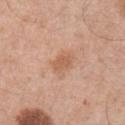Impression:
No biopsy was performed on this lesion — it was imaged during a full skin examination and was not determined to be concerning.
Background:
Imaged with white-light lighting. Automated image analysis of the tile measured a lesion area of about 3.5 mm², a shape eccentricity near 0.75, and a shape-asymmetry score of about 0.3 (0 = symmetric). The subject is a male aged around 70. A lesion tile, about 15 mm wide, cut from a 3D total-body photograph. From the abdomen.